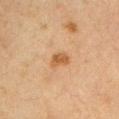<record>
<biopsy_status>not biopsied; imaged during a skin examination</biopsy_status>
<image>
  <source>total-body photography crop</source>
  <field_of_view_mm>15</field_of_view_mm>
</image>
<lesion_size>
  <long_diameter_mm_approx>2.5</long_diameter_mm_approx>
</lesion_size>
<site>right upper arm</site>
<automated_metrics>
  <area_mm2_approx>4.0</area_mm2_approx>
  <eccentricity>0.7</eccentricity>
  <nevus_likeness_0_100>85</nevus_likeness_0_100>
  <lesion_detection_confidence_0_100>100</lesion_detection_confidence_0_100>
</automated_metrics>
<patient>
  <sex>male</sex>
  <age_approx>65</age_approx>
</patient>
<lighting>cross-polarized</lighting>
</record>